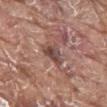Assessment:
Imaged during a routine full-body skin examination; the lesion was not biopsied and no histopathology is available.
Image and clinical context:
The lesion's longest dimension is about 3 mm. A 15 mm close-up extracted from a 3D total-body photography capture. This is a white-light tile. The lesion is located on the mid back. The patient is a male approximately 80 years of age.